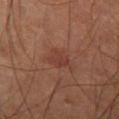| key | value |
|---|---|
| workup | catalogued during a skin exam; not biopsied |
| image-analysis metrics | a lesion area of about 5 mm²; a lesion color around L≈36 a*≈24 b*≈25 in CIELAB, about 6 CIELAB-L* units darker than the surrounding skin, and a normalized lesion–skin contrast near 5.5; an automated nevus-likeness rating near 15 out of 100 and a lesion-detection confidence of about 100/100 |
| imaging modality | total-body-photography crop, ~15 mm field of view |
| lesion diameter | ≈3 mm |
| lighting | cross-polarized illumination |
| body site | the right thigh |
| patient | male, aged around 65 |